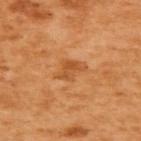Q: Was this lesion biopsied?
A: total-body-photography surveillance lesion; no biopsy
Q: What kind of image is this?
A: total-body-photography crop, ~15 mm field of view
Q: What lighting was used for the tile?
A: cross-polarized illumination
Q: How large is the lesion?
A: ≈3.5 mm
Q: Patient demographics?
A: female, approximately 55 years of age
Q: Lesion location?
A: the upper back